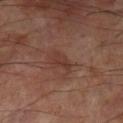biopsy status = total-body-photography surveillance lesion; no biopsy | body site = the left lower leg | diameter = ≈3 mm | lighting = cross-polarized illumination | acquisition = 15 mm crop, total-body photography | patient = male, aged approximately 70.